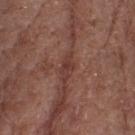<case>
<biopsy_status>not biopsied; imaged during a skin examination</biopsy_status>
<site>head or neck</site>
<image>
  <source>total-body photography crop</source>
  <field_of_view_mm>15</field_of_view_mm>
</image>
<lesion_size>
  <long_diameter_mm_approx>3.5</long_diameter_mm_approx>
</lesion_size>
<patient>
  <sex>female</sex>
  <age_approx>75</age_approx>
</patient>
<automated_metrics>
  <border_irregularity_0_10>3.5</border_irregularity_0_10>
  <peripheral_color_asymmetry>0.5</peripheral_color_asymmetry>
  <nevus_likeness_0_100>0</nevus_likeness_0_100>
  <lesion_detection_confidence_0_100>55</lesion_detection_confidence_0_100>
</automated_metrics>
</case>